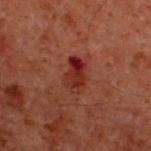| feature | finding |
|---|---|
| notes | no biopsy performed (imaged during a skin exam) |
| lighting | cross-polarized |
| acquisition | total-body-photography crop, ~15 mm field of view |
| site | the upper back |
| patient | male, aged 58–62 |